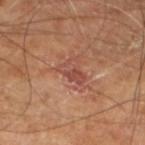biopsy status: no biopsy performed (imaged during a skin exam); tile lighting: cross-polarized; patient: male, approximately 65 years of age; location: the left lower leg; image: ~15 mm crop, total-body skin-cancer survey; lesion size: ≈3.5 mm.